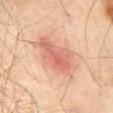biopsy_status: not biopsied; imaged during a skin examination
image:
  source: total-body photography crop
  field_of_view_mm: 15
lesion_size:
  long_diameter_mm_approx: 6.0
patient:
  sex: male
  age_approx: 70
lighting: cross-polarized
site: abdomen